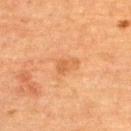follow-up — total-body-photography surveillance lesion; no biopsy | patient — female, about 70 years old | anatomic site — the upper back | image — total-body-photography crop, ~15 mm field of view.